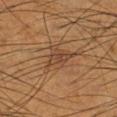Captured during whole-body skin photography for melanoma surveillance; the lesion was not biopsied. A 15 mm crop from a total-body photograph taken for skin-cancer surveillance. A male subject, aged 53–57. Measured at roughly 5 mm in maximum diameter. From the left lower leg.